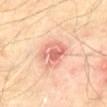Clinical impression:
No biopsy was performed on this lesion — it was imaged during a full skin examination and was not determined to be concerning.
Context:
A close-up tile cropped from a whole-body skin photograph, about 15 mm across. Captured under cross-polarized illumination. Automated image analysis of the tile measured a nevus-likeness score of about 10/100 and lesion-presence confidence of about 100/100. The lesion is on the lower back. The patient is a male aged around 60.